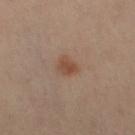Background: This is a cross-polarized tile. Longest diameter approximately 2.5 mm. A female subject approximately 40 years of age. On the left leg. A close-up tile cropped from a whole-body skin photograph, about 15 mm across.